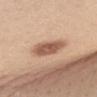Captured during whole-body skin photography for melanoma surveillance; the lesion was not biopsied. A female patient in their mid-40s. The total-body-photography lesion software estimated a footprint of about 9.5 mm² and a shape-asymmetry score of about 0.2 (0 = symmetric). And it measured a mean CIELAB color near L≈57 a*≈21 b*≈30, roughly 14 lightness units darker than nearby skin, and a normalized lesion–skin contrast near 8.5. It also reported a nevus-likeness score of about 90/100 and a detector confidence of about 100 out of 100 that the crop contains a lesion. This image is a 15 mm lesion crop taken from a total-body photograph. On the chest. About 4.5 mm across. Imaged with white-light lighting.